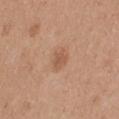notes — total-body-photography surveillance lesion; no biopsy | patient — female, aged 38–42 | anatomic site — the leg | image — 15 mm crop, total-body photography | size — ≈2.5 mm.